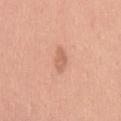The lesion was photographed on a routine skin check and not biopsied; there is no pathology result.
A male subject aged around 40.
Approximately 3.5 mm at its widest.
The total-body-photography lesion software estimated a lesion area of about 4.5 mm², an outline eccentricity of about 0.85 (0 = round, 1 = elongated), and two-axis asymmetry of about 0.3. It also reported lesion-presence confidence of about 100/100.
The lesion is located on the mid back.
A 15 mm close-up extracted from a 3D total-body photography capture.
Captured under white-light illumination.